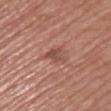Part of a total-body skin-imaging series; this lesion was reviewed on a skin check and was not flagged for biopsy. From the upper back. This is a white-light tile. About 2.5 mm across. A roughly 15 mm field-of-view crop from a total-body skin photograph. An algorithmic analysis of the crop reported a lesion area of about 4 mm², an outline eccentricity of about 0.6 (0 = round, 1 = elongated), and two-axis asymmetry of about 0.35. And it measured a lesion color around L≈49 a*≈24 b*≈26 in CIELAB and a lesion–skin lightness drop of about 9. The software also gave a classifier nevus-likeness of about 0/100 and a lesion-detection confidence of about 100/100. The patient is a female aged around 65.